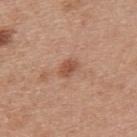Part of a total-body skin-imaging series; this lesion was reviewed on a skin check and was not flagged for biopsy.
A 15 mm close-up tile from a total-body photography series done for melanoma screening.
A male subject, aged 28–32.
On the upper back.